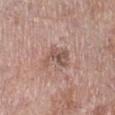The lesion was tiled from a total-body skin photograph and was not biopsied. Cropped from a whole-body photographic skin survey; the tile spans about 15 mm. A male patient aged around 75. Captured under white-light illumination. The recorded lesion diameter is about 3.5 mm. The lesion is located on the right lower leg.